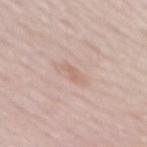Q: Was a biopsy performed?
A: total-body-photography surveillance lesion; no biopsy
Q: How large is the lesion?
A: ≈2.5 mm
Q: Lesion location?
A: the mid back
Q: How was this image acquired?
A: ~15 mm crop, total-body skin-cancer survey
Q: How was the tile lit?
A: white-light illumination
Q: What are the patient's age and sex?
A: female, about 50 years old